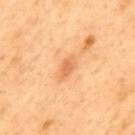Impression:
The lesion was tiled from a total-body skin photograph and was not biopsied.